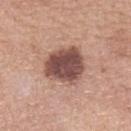Background:
A region of skin cropped from a whole-body photographic capture, roughly 15 mm wide. A female subject aged 58 to 62. Imaged with white-light lighting. About 5 mm across. On the arm. An algorithmic analysis of the crop reported a lesion area of about 17 mm² and two-axis asymmetry of about 0.2. And it measured radial color variation of about 1. The analysis additionally found lesion-presence confidence of about 100/100.